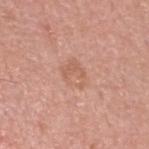Captured during whole-body skin photography for melanoma surveillance; the lesion was not biopsied. Approximately 3 mm at its widest. A male subject, aged around 55. An algorithmic analysis of the crop reported a lesion–skin lightness drop of about 6 and a lesion-to-skin contrast of about 5 (normalized; higher = more distinct). It also reported border irregularity of about 2.5 on a 0–10 scale and a within-lesion color-variation index near 3.5/10. The tile uses white-light illumination. The lesion is located on the head or neck. A region of skin cropped from a whole-body photographic capture, roughly 15 mm wide.